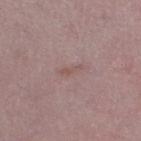Clinical summary: Captured under white-light illumination. The subject is a female roughly 45 years of age. A 15 mm close-up extracted from a 3D total-body photography capture. The lesion is on the right thigh.